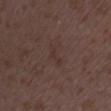This lesion was catalogued during total-body skin photography and was not selected for biopsy. The lesion is located on the right lower leg. A female patient aged 33 to 37. Cropped from a total-body skin-imaging series; the visible field is about 15 mm.